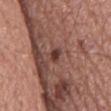<case>
<biopsy_status>not biopsied; imaged during a skin examination</biopsy_status>
<lesion_size>
  <long_diameter_mm_approx>2.5</long_diameter_mm_approx>
</lesion_size>
<automated_metrics>
  <nevus_likeness_0_100>15</nevus_likeness_0_100>
  <lesion_detection_confidence_0_100>100</lesion_detection_confidence_0_100>
</automated_metrics>
<patient>
  <sex>male</sex>
  <age_approx>75</age_approx>
</patient>
<site>mid back</site>
<lighting>white-light</lighting>
<image>
  <source>total-body photography crop</source>
  <field_of_view_mm>15</field_of_view_mm>
</image>
</case>